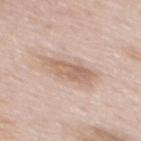Assessment: Recorded during total-body skin imaging; not selected for excision or biopsy. Acquisition and patient details: On the mid back. The subject is a male about 55 years old. A close-up tile cropped from a whole-body skin photograph, about 15 mm across.